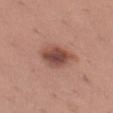Clinical impression: Part of a total-body skin-imaging series; this lesion was reviewed on a skin check and was not flagged for biopsy. Context: Measured at roughly 4 mm in maximum diameter. Located on the left thigh. The total-body-photography lesion software estimated two-axis asymmetry of about 0.2. The analysis additionally found a border-irregularity rating of about 2/10, a within-lesion color-variation index near 5/10, and radial color variation of about 1.5. A female patient, aged 28 to 32. Imaged with white-light lighting. A region of skin cropped from a whole-body photographic capture, roughly 15 mm wide.